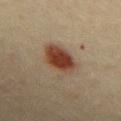{"biopsy_status": "not biopsied; imaged during a skin examination", "site": "mid back", "image": {"source": "total-body photography crop", "field_of_view_mm": 15}, "lesion_size": {"long_diameter_mm_approx": 4.5}, "patient": {"sex": "male", "age_approx": 40}}